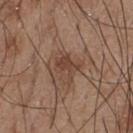Background: A male patient, in their mid- to late 50s. The total-body-photography lesion software estimated an average lesion color of about L≈42 a*≈19 b*≈26 (CIELAB) and a lesion–skin lightness drop of about 8. The software also gave a border-irregularity index near 5.5/10 and radial color variation of about 0.5. And it measured an automated nevus-likeness rating near 25 out of 100 and a detector confidence of about 100 out of 100 that the crop contains a lesion. The lesion is located on the chest. Cropped from a total-body skin-imaging series; the visible field is about 15 mm. The tile uses white-light illumination.